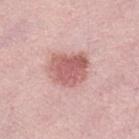Imaged during a routine full-body skin examination; the lesion was not biopsied and no histopathology is available.
Cropped from a total-body skin-imaging series; the visible field is about 15 mm.
The lesion is located on the left lower leg.
Automated tile analysis of the lesion measured an average lesion color of about L≈60 a*≈26 b*≈23 (CIELAB), a lesion–skin lightness drop of about 13, and a lesion-to-skin contrast of about 8 (normalized; higher = more distinct). The analysis additionally found an automated nevus-likeness rating near 90 out of 100 and lesion-presence confidence of about 100/100.
The subject is a female roughly 65 years of age.